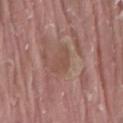Imaged during a routine full-body skin examination; the lesion was not biopsied and no histopathology is available.